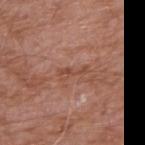Assessment:
No biopsy was performed on this lesion — it was imaged during a full skin examination and was not determined to be concerning.
Background:
The lesion is on the right upper arm. A male patient in their 60s. A region of skin cropped from a whole-body photographic capture, roughly 15 mm wide. About 3.5 mm across. This is a white-light tile.